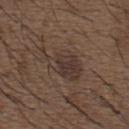subject: male, in their 50s | automated metrics: an eccentricity of roughly 0.85 and a symmetry-axis asymmetry near 0.4; a lesion–skin lightness drop of about 7 and a lesion-to-skin contrast of about 6.5 (normalized; higher = more distinct); a border-irregularity index near 4.5/10, a within-lesion color-variation index near 3.5/10, and radial color variation of about 1.5; a lesion-detection confidence of about 95/100 | anatomic site: the upper back | illumination: white-light illumination | lesion size: ~6 mm (longest diameter) | image source: ~15 mm tile from a whole-body skin photo.